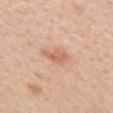follow-up = no biopsy performed (imaged during a skin exam); imaging modality = 15 mm crop, total-body photography; location = the mid back; subject = female, about 50 years old.